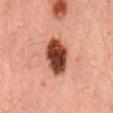The lesion was tiled from a total-body skin photograph and was not biopsied.
A male patient, in their 50s.
The lesion is on the mid back.
Cropped from a total-body skin-imaging series; the visible field is about 15 mm.